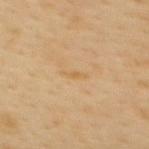acquisition: ~15 mm crop, total-body skin-cancer survey
patient: female, about 50 years old
location: the upper back